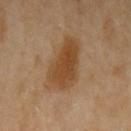No biopsy was performed on this lesion — it was imaged during a full skin examination and was not determined to be concerning. A female subject, in their 70s. Located on the left upper arm. The lesion's longest dimension is about 6.5 mm. A region of skin cropped from a whole-body photographic capture, roughly 15 mm wide. The tile uses cross-polarized illumination.